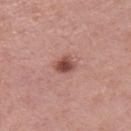Part of a total-body skin-imaging series; this lesion was reviewed on a skin check and was not flagged for biopsy. Measured at roughly 2.5 mm in maximum diameter. From the right thigh. A female patient in their 40s. Imaged with white-light lighting. Cropped from a total-body skin-imaging series; the visible field is about 15 mm.